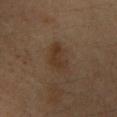- follow-up · imaged on a skin check; not biopsied
- location · the chest
- lesion size · ~3.5 mm (longest diameter)
- lighting · cross-polarized
- image-analysis metrics · a lesion area of about 8.5 mm² and an outline eccentricity of about 0.6 (0 = round, 1 = elongated); a mean CIELAB color near L≈30 a*≈14 b*≈25, about 6 CIELAB-L* units darker than the surrounding skin, and a normalized lesion–skin contrast near 6.5
- subject · male, about 35 years old
- acquisition · ~15 mm tile from a whole-body skin photo